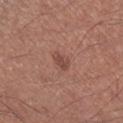Case summary:
• illumination · white-light illumination
• automated metrics · an area of roughly 3 mm²
• patient · male, in their 70s
• image source · total-body-photography crop, ~15 mm field of view
• diameter · ≈2.5 mm
• location · the right lower leg